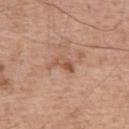  biopsy_status: not biopsied; imaged during a skin examination
  site: back
  automated_metrics:
    area_mm2_approx: 3.5
    eccentricity: 0.9
    border_irregularity_0_10: 4.0
    color_variation_0_10: 5.0
    peripheral_color_asymmetry: 1.5
    nevus_likeness_0_100: 0
  lesion_size:
    long_diameter_mm_approx: 3.0
  image:
    source: total-body photography crop
    field_of_view_mm: 15
  lighting: white-light
  patient:
    sex: male
    age_approx: 55